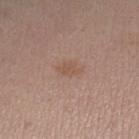Assessment:
Captured during whole-body skin photography for melanoma surveillance; the lesion was not biopsied.
Context:
This image is a 15 mm lesion crop taken from a total-body photograph. Located on the leg. A female patient, about 30 years old. Automated image analysis of the tile measured a footprint of about 4 mm², a shape eccentricity near 0.8, and a shape-asymmetry score of about 0.3 (0 = symmetric). The analysis additionally found internal color variation of about 1 on a 0–10 scale. And it measured a nevus-likeness score of about 0/100 and a detector confidence of about 100 out of 100 that the crop contains a lesion.